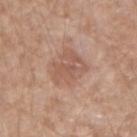Impression: Part of a total-body skin-imaging series; this lesion was reviewed on a skin check and was not flagged for biopsy. Acquisition and patient details: A 15 mm close-up tile from a total-body photography series done for melanoma screening. The patient is a male approximately 55 years of age. Located on the right upper arm.